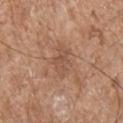Imaged during a routine full-body skin examination; the lesion was not biopsied and no histopathology is available. Cropped from a total-body skin-imaging series; the visible field is about 15 mm. The patient is a male aged around 70. This is a white-light tile. Measured at roughly 4 mm in maximum diameter. The lesion is located on the right upper arm.